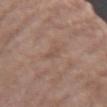Assessment: No biopsy was performed on this lesion — it was imaged during a full skin examination and was not determined to be concerning. Clinical summary: The lesion is located on the arm. Cropped from a whole-body photographic skin survey; the tile spans about 15 mm. A male patient aged 68–72. An algorithmic analysis of the crop reported a shape eccentricity near 0.95. It also reported a border-irregularity rating of about 5/10, a within-lesion color-variation index near 0/10, and a peripheral color-asymmetry measure near 0. The tile uses white-light illumination.